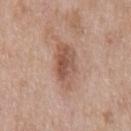The lesion was tiled from a total-body skin photograph and was not biopsied.